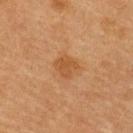biopsy status — imaged on a skin check; not biopsied | lesion size — about 2.5 mm | subject — female, aged 53–57 | image — ~15 mm tile from a whole-body skin photo | illumination — cross-polarized | site — the back | automated lesion analysis — an area of roughly 5.5 mm² and an eccentricity of roughly 0.45; a mean CIELAB color near L≈44 a*≈20 b*≈35, a lesion–skin lightness drop of about 7, and a normalized border contrast of about 6; a border-irregularity index near 2/10 and internal color variation of about 2 on a 0–10 scale; an automated nevus-likeness rating near 15 out of 100 and lesion-presence confidence of about 100/100.